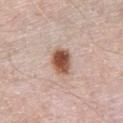| field | value |
|---|---|
| follow-up | no biopsy performed (imaged during a skin exam) |
| automated lesion analysis | an average lesion color of about L≈53 a*≈22 b*≈29 (CIELAB), a lesion–skin lightness drop of about 18, and a lesion-to-skin contrast of about 12 (normalized; higher = more distinct); a border-irregularity rating of about 1.5/10, a color-variation rating of about 5.5/10, and peripheral color asymmetry of about 1.5; an automated nevus-likeness rating near 100 out of 100 and lesion-presence confidence of about 100/100 |
| diameter | about 3.5 mm |
| location | the chest |
| illumination | white-light illumination |
| subject | male, approximately 80 years of age |
| image source | total-body-photography crop, ~15 mm field of view |